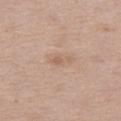Assessment: Captured during whole-body skin photography for melanoma surveillance; the lesion was not biopsied. Image and clinical context: The total-body-photography lesion software estimated a footprint of about 3 mm², an eccentricity of roughly 0.9, and a shape-asymmetry score of about 0.35 (0 = symmetric). It also reported a color-variation rating of about 1.5/10 and a peripheral color-asymmetry measure near 0.5. And it measured a nevus-likeness score of about 5/100 and a detector confidence of about 100 out of 100 that the crop contains a lesion. A female subject, aged 38–42. This is a white-light tile. A lesion tile, about 15 mm wide, cut from a 3D total-body photograph. The lesion's longest dimension is about 3 mm. Located on the left thigh.